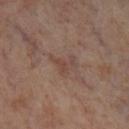{
  "biopsy_status": "not biopsied; imaged during a skin examination",
  "image": {
    "source": "total-body photography crop",
    "field_of_view_mm": 15
  },
  "automated_metrics": {
    "eccentricity": 0.75,
    "shape_asymmetry": 0.7,
    "nevus_likeness_0_100": 0,
    "lesion_detection_confidence_0_100": 95
  },
  "site": "leg",
  "patient": {
    "sex": "male",
    "age_approx": 70
  }
}